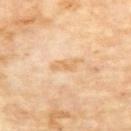Impression: This lesion was catalogued during total-body skin photography and was not selected for biopsy. Acquisition and patient details: The lesion-visualizer software estimated a lesion area of about 4 mm², a shape eccentricity near 0.9, and a symmetry-axis asymmetry near 0.45. The software also gave a lesion color around L≈72 a*≈19 b*≈42 in CIELAB, about 8 CIELAB-L* units darker than the surrounding skin, and a lesion-to-skin contrast of about 6 (normalized; higher = more distinct). It also reported a color-variation rating of about 1/10 and a peripheral color-asymmetry measure near 0. And it measured a lesion-detection confidence of about 100/100. This is a cross-polarized tile. This image is a 15 mm lesion crop taken from a total-body photograph. The lesion is located on the chest. A female subject, roughly 75 years of age.